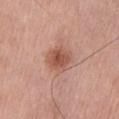<record>
  <biopsy_status>not biopsied; imaged during a skin examination</biopsy_status>
  <image>
    <source>total-body photography crop</source>
    <field_of_view_mm>15</field_of_view_mm>
  </image>
  <site>front of the torso</site>
  <lighting>white-light</lighting>
  <patient>
    <sex>male</sex>
    <age_approx>75</age_approx>
  </patient>
  <automated_metrics>
    <cielab_L>53</cielab_L>
    <cielab_a>24</cielab_a>
    <cielab_b>29</cielab_b>
    <vs_skin_contrast_norm>8.0</vs_skin_contrast_norm>
  </automated_metrics>
</record>